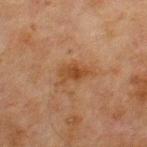Assessment: Imaged during a routine full-body skin examination; the lesion was not biopsied and no histopathology is available.